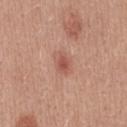| key | value |
|---|---|
| notes | no biopsy performed (imaged during a skin exam) |
| lighting | white-light illumination |
| acquisition | 15 mm crop, total-body photography |
| site | the chest |
| patient | male, approximately 25 years of age |
| diameter | ≈3.5 mm |
| automated metrics | a lesion-detection confidence of about 100/100 |